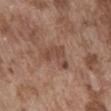Recorded during total-body skin imaging; not selected for excision or biopsy. This image is a 15 mm lesion crop taken from a total-body photograph. The patient is a male aged 73–77. The lesion's longest dimension is about 4 mm. Imaged with white-light lighting. The lesion is on the abdomen. Automated image analysis of the tile measured internal color variation of about 3.5 on a 0–10 scale.